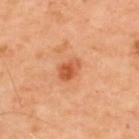Part of a total-body skin-imaging series; this lesion was reviewed on a skin check and was not flagged for biopsy.
The subject is a male aged 48–52.
Located on the upper back.
The recorded lesion diameter is about 3 mm.
Captured under cross-polarized illumination.
Cropped from a total-body skin-imaging series; the visible field is about 15 mm.